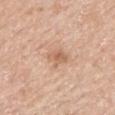Findings:
• notes · catalogued during a skin exam; not biopsied
• lighting · white-light illumination
• site · the mid back
• TBP lesion metrics · an area of roughly 4 mm², a shape eccentricity near 0.7, and a symmetry-axis asymmetry near 0.3; a lesion color around L≈62 a*≈21 b*≈33 in CIELAB and roughly 9 lightness units darker than nearby skin; a border-irregularity index near 3/10, internal color variation of about 2.5 on a 0–10 scale, and peripheral color asymmetry of about 0.5; a classifier nevus-likeness of about 10/100 and a lesion-detection confidence of about 100/100
• lesion size · about 2.5 mm
• acquisition · ~15 mm crop, total-body skin-cancer survey
• subject · male, in their 60s